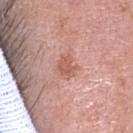The lesion was photographed on a routine skin check and not biopsied; there is no pathology result. The total-body-photography lesion software estimated a mean CIELAB color near L≈59 a*≈24 b*≈29, roughly 9 lightness units darker than nearby skin, and a lesion-to-skin contrast of about 6.5 (normalized; higher = more distinct). A female patient, approximately 40 years of age. From the head or neck. Cropped from a total-body skin-imaging series; the visible field is about 15 mm. The tile uses white-light illumination.